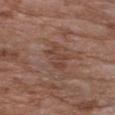This lesion was catalogued during total-body skin photography and was not selected for biopsy.
Captured under white-light illumination.
The lesion is on the chest.
A lesion tile, about 15 mm wide, cut from a 3D total-body photograph.
A female subject, roughly 75 years of age.